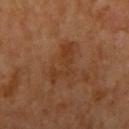biopsy_status: not biopsied; imaged during a skin examination
patient:
  sex: male
  age_approx: 60
lesion_size:
  long_diameter_mm_approx: 5.0
site: right upper arm
lighting: cross-polarized
image:
  source: total-body photography crop
  field_of_view_mm: 15
automated_metrics:
  border_irregularity_0_10: 8.0
  peripheral_color_asymmetry: 1.0
  nevus_likeness_0_100: 0
  lesion_detection_confidence_0_100: 100